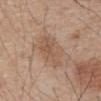<tbp_lesion>
  <image>
    <source>total-body photography crop</source>
    <field_of_view_mm>15</field_of_view_mm>
  </image>
  <lighting>white-light</lighting>
  <patient>
    <sex>male</sex>
    <age_approx>80</age_approx>
  </patient>
  <lesion_size>
    <long_diameter_mm_approx>5.0</long_diameter_mm_approx>
  </lesion_size>
  <site>abdomen</site>
</tbp_lesion>